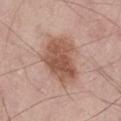This lesion was catalogued during total-body skin photography and was not selected for biopsy. Longest diameter approximately 6.5 mm. A 15 mm close-up tile from a total-body photography series done for melanoma screening. Located on the lower back. Imaged with white-light lighting. The lesion-visualizer software estimated a lesion color around L≈55 a*≈21 b*≈28 in CIELAB, about 12 CIELAB-L* units darker than the surrounding skin, and a normalized border contrast of about 8. It also reported an automated nevus-likeness rating near 85 out of 100 and a lesion-detection confidence of about 100/100. A male subject, about 65 years old.